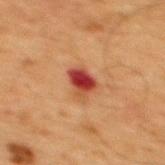A male subject, aged approximately 65.
Automated tile analysis of the lesion measured an average lesion color of about L≈38 a*≈29 b*≈30 (CIELAB), roughly 13 lightness units darker than nearby skin, and a normalized border contrast of about 10.5. The analysis additionally found a peripheral color-asymmetry measure near 3.
The lesion is located on the mid back.
A region of skin cropped from a whole-body photographic capture, roughly 15 mm wide.
The tile uses cross-polarized illumination.